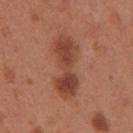The lesion was tiled from a total-body skin photograph and was not biopsied.
This is a white-light tile.
A female subject, about 30 years old.
About 7.5 mm across.
The lesion is on the arm.
Cropped from a total-body skin-imaging series; the visible field is about 15 mm.